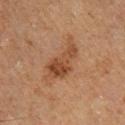• subject · male, aged 63 to 67
• lesion size · about 6.5 mm
• acquisition · ~15 mm crop, total-body skin-cancer survey
• site · the left lower leg
• lighting · cross-polarized